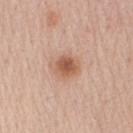A lesion tile, about 15 mm wide, cut from a 3D total-body photograph. A male subject, aged around 60. The recorded lesion diameter is about 3 mm. The lesion is on the right upper arm. Automated image analysis of the tile measured an outline eccentricity of about 0.45 (0 = round, 1 = elongated) and a shape-asymmetry score of about 0.2 (0 = symmetric). The analysis additionally found a border-irregularity rating of about 1.5/10 and a peripheral color-asymmetry measure near 1. This is a white-light tile.